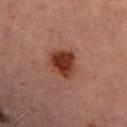{
  "lesion_size": {
    "long_diameter_mm_approx": 3.5
  },
  "patient": {
    "sex": "female",
    "age_approx": 70
  },
  "image": {
    "source": "total-body photography crop",
    "field_of_view_mm": 15
  },
  "lighting": "cross-polarized",
  "site": "leg"
}